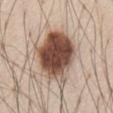biopsy status: imaged on a skin check; not biopsied | image-analysis metrics: an outline eccentricity of about 0.65 (0 = round, 1 = elongated) and a shape-asymmetry score of about 0.2 (0 = symmetric); a mean CIELAB color near L≈49 a*≈18 b*≈26; a border-irregularity index near 2.5/10, a color-variation rating of about 8.5/10, and peripheral color asymmetry of about 2.5; a classifier nevus-likeness of about 95/100 and a detector confidence of about 100 out of 100 that the crop contains a lesion | acquisition: ~15 mm crop, total-body skin-cancer survey | subject: male, in their 60s | site: the chest | illumination: white-light.